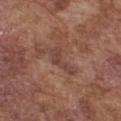{"image": {"source": "total-body photography crop", "field_of_view_mm": 15}, "automated_metrics": {"border_irregularity_0_10": 6.5, "color_variation_0_10": 0.5, "peripheral_color_asymmetry": 0.0, "lesion_detection_confidence_0_100": 60}, "patient": {"sex": "male", "age_approx": 75}, "site": "chest", "lesion_size": {"long_diameter_mm_approx": 4.0}}